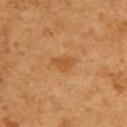This lesion was catalogued during total-body skin photography and was not selected for biopsy. Imaged with cross-polarized lighting. A 15 mm close-up extracted from a 3D total-body photography capture. The total-body-photography lesion software estimated a shape eccentricity near 0.8 and a symmetry-axis asymmetry near 0.35. The analysis additionally found a mean CIELAB color near L≈54 a*≈24 b*≈43. And it measured a border-irregularity rating of about 3/10 and a within-lesion color-variation index near 2/10. The recorded lesion diameter is about 3 mm. A female subject aged approximately 55. On the upper back.